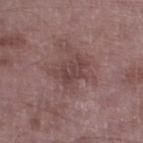Assessment:
The lesion was photographed on a routine skin check and not biopsied; there is no pathology result.
Image and clinical context:
A male patient, about 50 years old. This image is a 15 mm lesion crop taken from a total-body photograph. From the left lower leg. The tile uses white-light illumination.